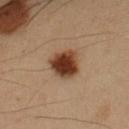Assessment: The lesion was tiled from a total-body skin photograph and was not biopsied. Image and clinical context: A roughly 15 mm field-of-view crop from a total-body skin photograph. From the arm. A male subject aged approximately 55. An algorithmic analysis of the crop reported an area of roughly 11 mm² and an eccentricity of roughly 0.65. It also reported an automated nevus-likeness rating near 100 out of 100 and a detector confidence of about 100 out of 100 that the crop contains a lesion.